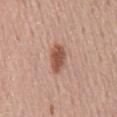follow-up: total-body-photography surveillance lesion; no biopsy
anatomic site: the mid back
diameter: ≈4 mm
automated lesion analysis: a mean CIELAB color near L≈53 a*≈23 b*≈28, a lesion–skin lightness drop of about 13, and a normalized border contrast of about 9; a border-irregularity index near 2/10 and internal color variation of about 3.5 on a 0–10 scale; an automated nevus-likeness rating near 95 out of 100
subject: male, roughly 55 years of age
acquisition: 15 mm crop, total-body photography